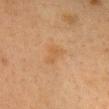Findings:
– workup — no biopsy performed (imaged during a skin exam)
– site — the head or neck
– image source — ~15 mm crop, total-body skin-cancer survey
– patient — female, aged approximately 40
– lighting — cross-polarized
– automated metrics — a footprint of about 4 mm², an eccentricity of roughly 0.7, and two-axis asymmetry of about 0.35; an automated nevus-likeness rating near 0 out of 100 and lesion-presence confidence of about 100/100